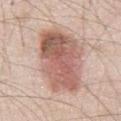Findings:
- notes: imaged on a skin check; not biopsied
- lesion diameter: ~9.5 mm (longest diameter)
- imaging modality: 15 mm crop, total-body photography
- illumination: white-light illumination
- body site: the abdomen
- subject: male, aged around 45